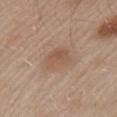The lesion was tiled from a total-body skin photograph and was not biopsied. A 15 mm close-up extracted from a 3D total-body photography capture. This is a white-light tile. The lesion is on the left upper arm. Measured at roughly 3.5 mm in maximum diameter. A male subject aged approximately 55.